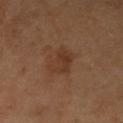notes — total-body-photography surveillance lesion; no biopsy
lesion size — ~3.5 mm (longest diameter)
automated metrics — an average lesion color of about L≈36 a*≈20 b*≈30 (CIELAB); a classifier nevus-likeness of about 40/100 and lesion-presence confidence of about 100/100
patient — female, aged 53–57
site — the left forearm
acquisition — ~15 mm crop, total-body skin-cancer survey
lighting — cross-polarized illumination